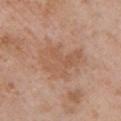Impression:
No biopsy was performed on this lesion — it was imaged during a full skin examination and was not determined to be concerning.
Acquisition and patient details:
A close-up tile cropped from a whole-body skin photograph, about 15 mm across. On the chest. A female subject, aged around 40.